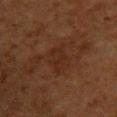workup: imaged on a skin check; not biopsied
TBP lesion metrics: an area of roughly 5 mm², an outline eccentricity of about 0.65 (0 = round, 1 = elongated), and a shape-asymmetry score of about 0.3 (0 = symmetric); a lesion color around L≈22 a*≈18 b*≈23 in CIELAB and about 4 CIELAB-L* units darker than the surrounding skin; a classifier nevus-likeness of about 0/100 and a detector confidence of about 100 out of 100 that the crop contains a lesion
image: total-body-photography crop, ~15 mm field of view
lighting: cross-polarized
site: the upper back
subject: female, roughly 50 years of age